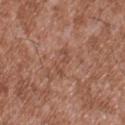Clinical summary: A roughly 15 mm field-of-view crop from a total-body skin photograph. Located on the upper back. This is a white-light tile. The patient is a male roughly 45 years of age.